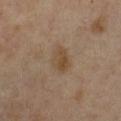On the chest.
A female subject aged 43 to 47.
A close-up tile cropped from a whole-body skin photograph, about 15 mm across.